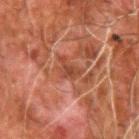- follow-up: total-body-photography surveillance lesion; no biopsy
- image: total-body-photography crop, ~15 mm field of view
- patient: male, aged 78–82
- location: the arm